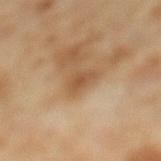Clinical impression:
Imaged during a routine full-body skin examination; the lesion was not biopsied and no histopathology is available.
Image and clinical context:
The lesion is located on the left lower leg. A lesion tile, about 15 mm wide, cut from a 3D total-body photograph. The subject is a female aged 58–62.